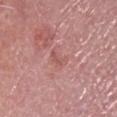Captured during whole-body skin photography for melanoma surveillance; the lesion was not biopsied. A 15 mm close-up tile from a total-body photography series done for melanoma screening. The lesion is located on the head or neck. Approximately 2.5 mm at its widest. Captured under white-light illumination. A male subject in their mid- to late 80s or older.